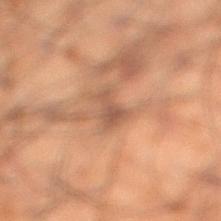Findings:
• biopsy status · imaged on a skin check; not biopsied
• lighting · cross-polarized
• acquisition · 15 mm crop, total-body photography
• patient · male, aged 48–52
• lesion diameter · ≈2.5 mm
• location · the right lower leg